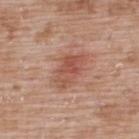* biopsy status · no biopsy performed (imaged during a skin exam)
* image · ~15 mm crop, total-body skin-cancer survey
* lighting · white-light illumination
* TBP lesion metrics · a footprint of about 9 mm² and an eccentricity of roughly 0.8; a lesion color around L≈53 a*≈25 b*≈29 in CIELAB, roughly 9 lightness units darker than nearby skin, and a normalized lesion–skin contrast near 6.5; a border-irregularity index near 3/10, a color-variation rating of about 5/10, and radial color variation of about 1.5
* size · ≈4.5 mm
* subject · male, in their 50s
* site · the upper back